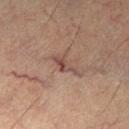No biopsy was performed on this lesion — it was imaged during a full skin examination and was not determined to be concerning.
The patient is a male roughly 60 years of age.
An algorithmic analysis of the crop reported a footprint of about 3.5 mm² and a symmetry-axis asymmetry near 0.45. And it measured a border-irregularity rating of about 5.5/10, a color-variation rating of about 2.5/10, and a peripheral color-asymmetry measure near 1.
A roughly 15 mm field-of-view crop from a total-body skin photograph.
On the left thigh.
The tile uses cross-polarized illumination.
About 3.5 mm across.